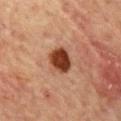No biopsy was performed on this lesion — it was imaged during a full skin examination and was not determined to be concerning. The lesion is located on the mid back. The subject is a male in their mid-60s. A 15 mm crop from a total-body photograph taken for skin-cancer surveillance.